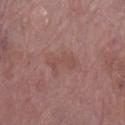{"biopsy_status": "not biopsied; imaged during a skin examination", "image": {"source": "total-body photography crop", "field_of_view_mm": 15}, "patient": {"sex": "female", "age_approx": 70}, "site": "right lower leg"}